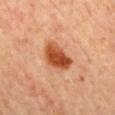biopsy status = imaged on a skin check; not biopsied | location = the back | patient = male, aged around 55 | image source = ~15 mm crop, total-body skin-cancer survey | tile lighting = cross-polarized.